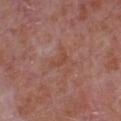biopsy_status: not biopsied; imaged during a skin examination
lesion_size:
  long_diameter_mm_approx: 2.5
site: front of the torso
patient:
  sex: male
  age_approx: 65
lighting: white-light
image:
  source: total-body photography crop
  field_of_view_mm: 15